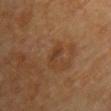<lesion>
  <biopsy_status>not biopsied; imaged during a skin examination</biopsy_status>
  <lighting>cross-polarized</lighting>
  <site>chest</site>
  <automated_metrics>
    <area_mm2_approx>2.5</area_mm2_approx>
    <shape_asymmetry>0.25</shape_asymmetry>
    <cielab_L>32</cielab_L>
    <cielab_a>17</cielab_a>
    <cielab_b>28</cielab_b>
    <vs_skin_darker_L>5.0</vs_skin_darker_L>
    <vs_skin_contrast_norm>5.5</vs_skin_contrast_norm>
    <nevus_likeness_0_100>0</nevus_likeness_0_100>
  </automated_metrics>
  <image>
    <source>total-body photography crop</source>
    <field_of_view_mm>15</field_of_view_mm>
  </image>
  <patient>
    <sex>female</sex>
    <age_approx>80</age_approx>
  </patient>
  <lesion_size>
    <long_diameter_mm_approx>3.0</long_diameter_mm_approx>
  </lesion_size>
</lesion>